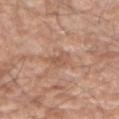biopsy_status: not biopsied; imaged during a skin examination
site: arm
lesion_size:
  long_diameter_mm_approx: 3.0
image:
  source: total-body photography crop
  field_of_view_mm: 15
lighting: white-light
patient:
  sex: male
  age_approx: 60